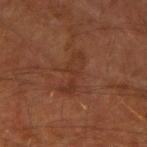Q: Was a biopsy performed?
A: total-body-photography surveillance lesion; no biopsy
Q: What kind of image is this?
A: ~15 mm tile from a whole-body skin photo
Q: Lesion location?
A: the right upper arm
Q: What are the patient's age and sex?
A: male, in their 60s